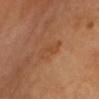Findings:
- biopsy status · catalogued during a skin exam; not biopsied
- image-analysis metrics · a lesion color around L≈45 a*≈22 b*≈34 in CIELAB, about 5 CIELAB-L* units darker than the surrounding skin, and a normalized border contrast of about 4.5; a border-irregularity rating of about 4.5/10 and a within-lesion color-variation index near 1.5/10; an automated nevus-likeness rating near 0 out of 100 and a lesion-detection confidence of about 100/100
- illumination · cross-polarized illumination
- size · about 3.5 mm
- patient · female, aged 63 to 67
- anatomic site · the head or neck
- image source · ~15 mm tile from a whole-body skin photo